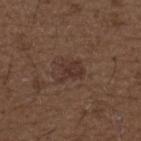The lesion was tiled from a total-body skin photograph and was not biopsied. On the upper back. A male subject in their 50s. Cropped from a total-body skin-imaging series; the visible field is about 15 mm. Captured under white-light illumination.